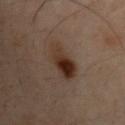Captured during whole-body skin photography for melanoma surveillance; the lesion was not biopsied. Automated image analysis of the tile measured an automated nevus-likeness rating near 100 out of 100. The tile uses cross-polarized illumination. A region of skin cropped from a whole-body photographic capture, roughly 15 mm wide. From the chest. About 4 mm across. The patient is a male in their 30s.